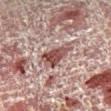Part of a total-body skin-imaging series; this lesion was reviewed on a skin check and was not flagged for biopsy. Approximately 4.5 mm at its widest. Imaged with cross-polarized lighting. A 15 mm close-up extracted from a 3D total-body photography capture. A male subject aged 53–57. Automated tile analysis of the lesion measured an area of roughly 9.5 mm². It also reported border irregularity of about 4.5 on a 0–10 scale, a color-variation rating of about 5.5/10, and radial color variation of about 1.5. It also reported a classifier nevus-likeness of about 0/100 and a detector confidence of about 75 out of 100 that the crop contains a lesion. The lesion is located on the right lower leg.